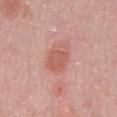Captured during whole-body skin photography for melanoma surveillance; the lesion was not biopsied. Cropped from a whole-body photographic skin survey; the tile spans about 15 mm. A male patient approximately 50 years of age. The lesion is on the abdomen.